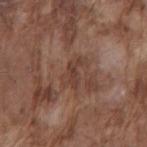workup: no biopsy performed (imaged during a skin exam)
size: about 3.5 mm
automated lesion analysis: an area of roughly 5.5 mm² and a shape eccentricity near 0.85; border irregularity of about 5.5 on a 0–10 scale, a color-variation rating of about 2/10, and a peripheral color-asymmetry measure near 0.5; a nevus-likeness score of about 0/100 and a detector confidence of about 95 out of 100 that the crop contains a lesion
body site: the mid back
acquisition: ~15 mm tile from a whole-body skin photo
subject: male, in their mid-70s
tile lighting: white-light illumination